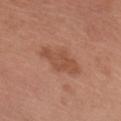Captured during whole-body skin photography for melanoma surveillance; the lesion was not biopsied. Longest diameter approximately 5 mm. A close-up tile cropped from a whole-body skin photograph, about 15 mm across. An algorithmic analysis of the crop reported an average lesion color of about L≈50 a*≈24 b*≈31 (CIELAB), a lesion–skin lightness drop of about 8, and a normalized border contrast of about 6. The analysis additionally found an automated nevus-likeness rating near 40 out of 100 and a detector confidence of about 100 out of 100 that the crop contains a lesion. A female subject aged 63–67. This is a white-light tile. The lesion is on the left forearm.